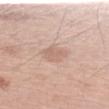Assessment:
The lesion was tiled from a total-body skin photograph and was not biopsied.
Acquisition and patient details:
This is a white-light tile. The subject is a male approximately 60 years of age. From the left forearm. The lesion-visualizer software estimated a footprint of about 6.5 mm² and a symmetry-axis asymmetry near 0.2. It also reported a border-irregularity index near 2/10 and peripheral color asymmetry of about 0.5. Approximately 3.5 mm at its widest. A 15 mm close-up extracted from a 3D total-body photography capture.